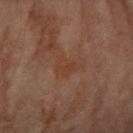This lesion was catalogued during total-body skin photography and was not selected for biopsy. Automated tile analysis of the lesion measured a lesion area of about 5 mm², an outline eccentricity of about 0.6 (0 = round, 1 = elongated), and a symmetry-axis asymmetry near 0.35. And it measured about 4 CIELAB-L* units darker than the surrounding skin and a lesion-to-skin contrast of about 4.5 (normalized; higher = more distinct). And it measured border irregularity of about 3 on a 0–10 scale, a within-lesion color-variation index near 2/10, and a peripheral color-asymmetry measure near 0.5. The analysis additionally found a nevus-likeness score of about 0/100 and a detector confidence of about 100 out of 100 that the crop contains a lesion. The recorded lesion diameter is about 3 mm. A 15 mm close-up tile from a total-body photography series done for melanoma screening. From the right forearm. This is a cross-polarized tile. A female subject aged 78 to 82.